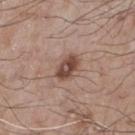Findings:
• notes — imaged on a skin check; not biopsied
• illumination — white-light illumination
• patient — male, roughly 75 years of age
• lesion diameter — ≈3.5 mm
• acquisition — total-body-photography crop, ~15 mm field of view
• site — the chest
• automated metrics — a mean CIELAB color near L≈47 a*≈19 b*≈25 and a normalized lesion–skin contrast near 9.5; a detector confidence of about 100 out of 100 that the crop contains a lesion